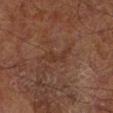Assessment:
Imaged during a routine full-body skin examination; the lesion was not biopsied and no histopathology is available.
Image and clinical context:
An algorithmic analysis of the crop reported a symmetry-axis asymmetry near 0.6. The software also gave radial color variation of about 0. The software also gave a classifier nevus-likeness of about 0/100 and lesion-presence confidence of about 85/100. The lesion is on the leg. About 4.5 mm across. A 15 mm crop from a total-body photograph taken for skin-cancer surveillance. The patient is a male roughly 65 years of age.